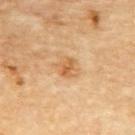{"image": {"source": "total-body photography crop", "field_of_view_mm": 15}, "patient": {"sex": "male", "age_approx": "85+"}, "site": "back"}